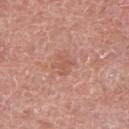A roughly 15 mm field-of-view crop from a total-body skin photograph. A female subject in their 60s. The lesion is located on the right upper arm.